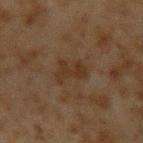acquisition — 15 mm crop, total-body photography; patient — male, aged approximately 45; location — the left upper arm.